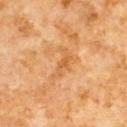Impression:
The lesion was photographed on a routine skin check and not biopsied; there is no pathology result.
Background:
A region of skin cropped from a whole-body photographic capture, roughly 15 mm wide. Automated image analysis of the tile measured an average lesion color of about L≈59 a*≈25 b*≈44 (CIELAB), a lesion–skin lightness drop of about 7, and a normalized lesion–skin contrast near 5.5. And it measured a border-irregularity rating of about 8/10 and internal color variation of about 2 on a 0–10 scale. This is a cross-polarized tile. Approximately 4 mm at its widest. The lesion is located on the chest. A male subject about 60 years old.